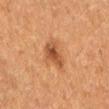Findings:
- workup: imaged on a skin check; not biopsied
- lesion size: ~3.5 mm (longest diameter)
- illumination: cross-polarized illumination
- location: the lower back
- imaging modality: 15 mm crop, total-body photography
- subject: female, in their 50s